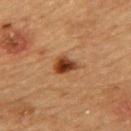Clinical impression: The lesion was photographed on a routine skin check and not biopsied; there is no pathology result. Background: A roughly 15 mm field-of-view crop from a total-body skin photograph. The tile uses cross-polarized illumination. The lesion is on the upper back. A female subject roughly 70 years of age. Longest diameter approximately 2.5 mm. An algorithmic analysis of the crop reported a lesion color around L≈34 a*≈22 b*≈31 in CIELAB, a lesion–skin lightness drop of about 14, and a normalized border contrast of about 12. It also reported a border-irregularity rating of about 2.5/10, a color-variation rating of about 6.5/10, and peripheral color asymmetry of about 2.